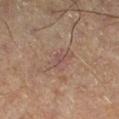automated_metrics:
  border_irregularity_0_10: 5.5
  color_variation_0_10: 0.0
patient:
  sex: male
  age_approx: 65
image:
  source: total-body photography crop
  field_of_view_mm: 15
site: left lower leg
lighting: cross-polarized